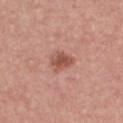Clinical impression: Imaged during a routine full-body skin examination; the lesion was not biopsied and no histopathology is available. Acquisition and patient details: From the chest. A male subject aged 58 to 62. A lesion tile, about 15 mm wide, cut from a 3D total-body photograph.